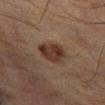biopsy status: imaged on a skin check; not biopsied
patient: male, aged approximately 65
body site: the leg
illumination: cross-polarized illumination
acquisition: ~15 mm tile from a whole-body skin photo
lesion diameter: ≈3.5 mm
image-analysis metrics: a footprint of about 8 mm² and a shape-asymmetry score of about 0.2 (0 = symmetric); a lesion color around L≈28 a*≈16 b*≈23 in CIELAB, roughly 10 lightness units darker than nearby skin, and a normalized border contrast of about 10